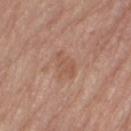The lesion was tiled from a total-body skin photograph and was not biopsied.